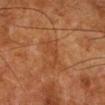Recorded during total-body skin imaging; not selected for excision or biopsy.
From the right lower leg.
A 15 mm close-up extracted from a 3D total-body photography capture.
A male patient approximately 80 years of age.